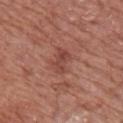  biopsy_status: not biopsied; imaged during a skin examination
  lighting: white-light
  lesion_size:
    long_diameter_mm_approx: 3.0
  image:
    source: total-body photography crop
    field_of_view_mm: 15
  site: arm
  automated_metrics:
    area_mm2_approx: 5.5
    eccentricity: 0.8
    shape_asymmetry: 0.3
    cielab_L: 45
    cielab_a: 26
    cielab_b: 27
    vs_skin_contrast_norm: 6.0
    color_variation_0_10: 3.5
    nevus_likeness_0_100: 0
    lesion_detection_confidence_0_100: 100
  patient:
    sex: male
    age_approx: 75